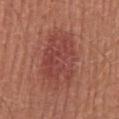Captured during whole-body skin photography for melanoma surveillance; the lesion was not biopsied.
A male patient roughly 45 years of age.
A close-up tile cropped from a whole-body skin photograph, about 15 mm across.
The lesion is located on the abdomen.